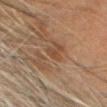notes = no biopsy performed (imaged during a skin exam)
image = total-body-photography crop, ~15 mm field of view
location = the head or neck
subject = male, aged 58 to 62